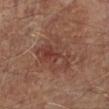Captured during whole-body skin photography for melanoma surveillance; the lesion was not biopsied. A male patient, aged 63–67. The lesion is located on the left lower leg. About 5.5 mm across. The lesion-visualizer software estimated a border-irregularity rating of about 3/10, internal color variation of about 5.5 on a 0–10 scale, and peripheral color asymmetry of about 2. It also reported an automated nevus-likeness rating near 5 out of 100 and lesion-presence confidence of about 100/100. Imaged with cross-polarized lighting. A roughly 15 mm field-of-view crop from a total-body skin photograph.